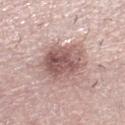workup: imaged on a skin check; not biopsied | subject: female, in their 40s | anatomic site: the right lower leg | size: about 6 mm | lighting: white-light | image: ~15 mm tile from a whole-body skin photo.